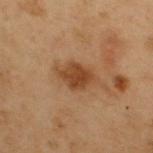{"biopsy_status": "not biopsied; imaged during a skin examination", "lighting": "cross-polarized", "patient": {"sex": "male", "age_approx": 55}, "image": {"source": "total-body photography crop", "field_of_view_mm": 15}, "automated_metrics": {"area_mm2_approx": 8.0, "shape_asymmetry": 0.2, "nevus_likeness_0_100": 55, "lesion_detection_confidence_0_100": 100}, "lesion_size": {"long_diameter_mm_approx": 4.5}, "site": "upper back"}